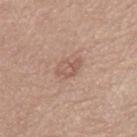Q: Is there a histopathology result?
A: imaged on a skin check; not biopsied
Q: Patient demographics?
A: female, aged approximately 65
Q: What kind of image is this?
A: 15 mm crop, total-body photography
Q: What is the anatomic site?
A: the leg